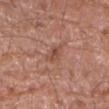| field | value |
|---|---|
| TBP lesion metrics | an outline eccentricity of about 0.85 (0 = round, 1 = elongated) and a shape-asymmetry score of about 0.4 (0 = symmetric); an average lesion color of about L≈49 a*≈23 b*≈29 (CIELAB), about 8 CIELAB-L* units darker than the surrounding skin, and a lesion-to-skin contrast of about 6 (normalized; higher = more distinct); radial color variation of about 1.5; a classifier nevus-likeness of about 0/100 and a detector confidence of about 100 out of 100 that the crop contains a lesion |
| image | ~15 mm crop, total-body skin-cancer survey |
| subject | male, approximately 80 years of age |
| lesion size | ~3 mm (longest diameter) |
| location | the right forearm |